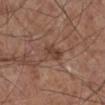<case>
  <biopsy_status>not biopsied; imaged during a skin examination</biopsy_status>
  <patient>
    <sex>male</sex>
    <age_approx>60</age_approx>
  </patient>
  <site>right lower leg</site>
  <image>
    <source>total-body photography crop</source>
    <field_of_view_mm>15</field_of_view_mm>
  </image>
</case>